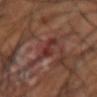notes = no biopsy performed (imaged during a skin exam) | lighting = cross-polarized | image = ~15 mm tile from a whole-body skin photo | TBP lesion metrics = a nevus-likeness score of about 0/100 | patient = male, aged approximately 40 | location = the right forearm.